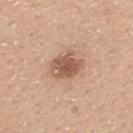| feature | finding |
|---|---|
| notes | no biopsy performed (imaged during a skin exam) |
| lighting | white-light |
| body site | the back |
| diameter | ≈4 mm |
| acquisition | ~15 mm tile from a whole-body skin photo |
| subject | male, aged approximately 30 |